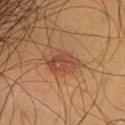Impression:
Part of a total-body skin-imaging series; this lesion was reviewed on a skin check and was not flagged for biopsy.
Background:
The lesion is on the chest. The subject is a male aged 58 to 62. Captured under cross-polarized illumination. A lesion tile, about 15 mm wide, cut from a 3D total-body photograph. The recorded lesion diameter is about 3.5 mm. Automated image analysis of the tile measured a border-irregularity rating of about 2.5/10, internal color variation of about 3.5 on a 0–10 scale, and peripheral color asymmetry of about 1.5.